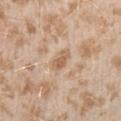<case>
  <biopsy_status>not biopsied; imaged during a skin examination</biopsy_status>
  <lighting>white-light</lighting>
  <patient>
    <sex>female</sex>
    <age_approx>25</age_approx>
  </patient>
  <lesion_size>
    <long_diameter_mm_approx>3.0</long_diameter_mm_approx>
  </lesion_size>
  <image>
    <source>total-body photography crop</source>
    <field_of_view_mm>15</field_of_view_mm>
  </image>
  <automated_metrics>
    <cielab_L>61</cielab_L>
    <cielab_a>17</cielab_a>
    <cielab_b>33</cielab_b>
    <vs_skin_darker_L>10.0</vs_skin_darker_L>
    <vs_skin_contrast_norm>7.0</vs_skin_contrast_norm>
    <border_irregularity_0_10>3.5</border_irregularity_0_10>
    <color_variation_0_10>1.5</color_variation_0_10>
    <peripheral_color_asymmetry>0.5</peripheral_color_asymmetry>
    <nevus_likeness_0_100>0</nevus_likeness_0_100>
    <lesion_detection_confidence_0_100>95</lesion_detection_confidence_0_100>
  </automated_metrics>
  <site>left upper arm</site>
</case>